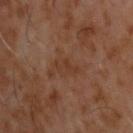The lesion was photographed on a routine skin check and not biopsied; there is no pathology result.
Located on the back.
The patient is a male roughly 60 years of age.
Cropped from a total-body skin-imaging series; the visible field is about 15 mm.
The tile uses cross-polarized illumination.